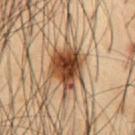Assessment: Captured during whole-body skin photography for melanoma surveillance; the lesion was not biopsied. Acquisition and patient details: The patient is a male aged 53–57. A lesion tile, about 15 mm wide, cut from a 3D total-body photograph. Longest diameter approximately 5.5 mm. The lesion is located on the abdomen.